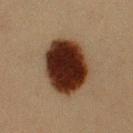Clinical impression: This lesion was catalogued during total-body skin photography and was not selected for biopsy. Image and clinical context: A 15 mm crop from a total-body photograph taken for skin-cancer surveillance. A male subject, in their 40s. The lesion is on the arm.